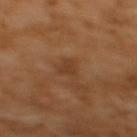follow-up: imaged on a skin check; not biopsied
illumination: cross-polarized
lesion size: ~2.5 mm (longest diameter)
patient: female, approximately 55 years of age
image source: ~15 mm tile from a whole-body skin photo
body site: the upper back